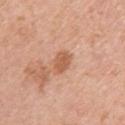Captured during whole-body skin photography for melanoma surveillance; the lesion was not biopsied.